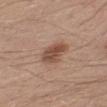The lesion was tiled from a total-body skin photograph and was not biopsied. This is a white-light tile. A male patient about 30 years old. Measured at roughly 4.5 mm in maximum diameter. This image is a 15 mm lesion crop taken from a total-body photograph. The lesion is on the left lower leg. Automated image analysis of the tile measured a lesion area of about 7.5 mm², a shape eccentricity near 0.8, and a shape-asymmetry score of about 0.25 (0 = symmetric). The analysis additionally found a classifier nevus-likeness of about 85/100 and a lesion-detection confidence of about 100/100.